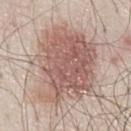The lesion was tiled from a total-body skin photograph and was not biopsied. Captured under white-light illumination. Longest diameter approximately 9.5 mm. A male patient, aged 78 to 82. On the chest. The total-body-photography lesion software estimated a lesion color around L≈58 a*≈18 b*≈24 in CIELAB and about 12 CIELAB-L* units darker than the surrounding skin. It also reported border irregularity of about 4 on a 0–10 scale and internal color variation of about 5 on a 0–10 scale. The analysis additionally found an automated nevus-likeness rating near 95 out of 100 and a lesion-detection confidence of about 95/100. A 15 mm crop from a total-body photograph taken for skin-cancer surveillance.